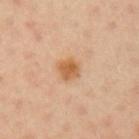biopsy status: imaged on a skin check; not biopsied
image source: ~15 mm tile from a whole-body skin photo
lesion size: about 2.5 mm
anatomic site: the left upper arm
patient: male, roughly 40 years of age